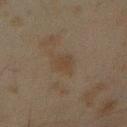Imaged during a routine full-body skin examination; the lesion was not biopsied and no histopathology is available. A region of skin cropped from a whole-body photographic capture, roughly 15 mm wide. A male patient approximately 45 years of age. The lesion's longest dimension is about 3.5 mm. The tile uses cross-polarized illumination. From the right forearm. The total-body-photography lesion software estimated a lesion area of about 6 mm² and two-axis asymmetry of about 0.2. The analysis additionally found a nevus-likeness score of about 0/100 and lesion-presence confidence of about 100/100.